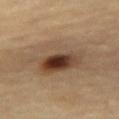Findings:
• notes — imaged on a skin check; not biopsied
• patient — female, aged around 70
• image — ~15 mm tile from a whole-body skin photo
• anatomic site — the right thigh